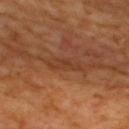Imaged during a routine full-body skin examination; the lesion was not biopsied and no histopathology is available.
The patient is a male aged 63 to 67.
Imaged with cross-polarized lighting.
A lesion tile, about 15 mm wide, cut from a 3D total-body photograph.
From the upper back.
The recorded lesion diameter is about 4 mm.